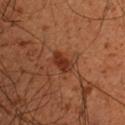The tile uses cross-polarized illumination. Automated image analysis of the tile measured an area of roughly 4.5 mm², an outline eccentricity of about 0.8 (0 = round, 1 = elongated), and a symmetry-axis asymmetry near 0.25. The software also gave a mean CIELAB color near L≈32 a*≈26 b*≈31 and a lesion-to-skin contrast of about 8.5 (normalized; higher = more distinct). And it measured a border-irregularity index near 2.5/10, a within-lesion color-variation index near 1.5/10, and a peripheral color-asymmetry measure near 0.5. On the chest. About 3 mm across. The subject is a male roughly 50 years of age. A close-up tile cropped from a whole-body skin photograph, about 15 mm across.